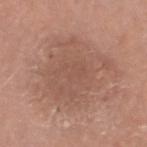Impression:
No biopsy was performed on this lesion — it was imaged during a full skin examination and was not determined to be concerning.
Context:
The lesion is located on the head or neck. A roughly 15 mm field-of-view crop from a total-body skin photograph. A male subject in their 60s. This is a white-light tile.